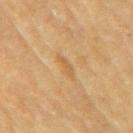biopsy_status: not biopsied; imaged during a skin examination
image:
  source: total-body photography crop
  field_of_view_mm: 15
lighting: cross-polarized
site: right upper arm
patient:
  sex: male
  age_approx: 85+
automated_metrics:
  area_mm2_approx: 2.5
  eccentricity: 0.9
  shape_asymmetry: 0.35
  cielab_L: 51
  cielab_a: 16
  cielab_b: 36
  vs_skin_darker_L: 6.0
  vs_skin_contrast_norm: 5.0
  nevus_likeness_0_100: 0
lesion_size:
  long_diameter_mm_approx: 2.5